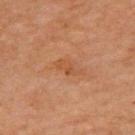* follow-up · imaged on a skin check; not biopsied
* image · total-body-photography crop, ~15 mm field of view
* subject · female, about 70 years old
* site · the upper back